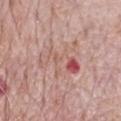Q: Was a biopsy performed?
A: imaged on a skin check; not biopsied
Q: Illumination type?
A: white-light
Q: What is the imaging modality?
A: 15 mm crop, total-body photography
Q: Who is the patient?
A: male, aged around 70
Q: How large is the lesion?
A: ~5.5 mm (longest diameter)
Q: Where on the body is the lesion?
A: the chest